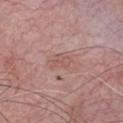<case>
  <biopsy_status>not biopsied; imaged during a skin examination</biopsy_status>
  <site>chest</site>
  <lesion_size>
    <long_diameter_mm_approx>3.0</long_diameter_mm_approx>
  </lesion_size>
  <image>
    <source>total-body photography crop</source>
    <field_of_view_mm>15</field_of_view_mm>
  </image>
  <lighting>white-light</lighting>
  <patient>
    <sex>male</sex>
    <age_approx>60</age_approx>
  </patient>
  <automated_metrics>
    <cielab_L>55</cielab_L>
    <cielab_a>22</cielab_a>
    <cielab_b>24</cielab_b>
    <vs_skin_contrast_norm>5.0</vs_skin_contrast_norm>
    <nevus_likeness_0_100>0</nevus_likeness_0_100>
    <lesion_detection_confidence_0_100>95</lesion_detection_confidence_0_100>
  </automated_metrics>
</case>